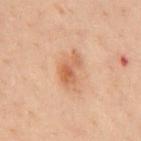{"biopsy_status": "not biopsied; imaged during a skin examination", "automated_metrics": {"vs_skin_darker_L": 8.0, "vs_skin_contrast_norm": 6.5, "nevus_likeness_0_100": 60, "lesion_detection_confidence_0_100": 100}, "site": "chest", "lighting": "cross-polarized", "lesion_size": {"long_diameter_mm_approx": 4.0}, "image": {"source": "total-body photography crop", "field_of_view_mm": 15}, "patient": {"sex": "male", "age_approx": 40}}